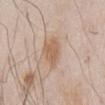Q: Was a biopsy performed?
A: catalogued during a skin exam; not biopsied
Q: What did automated image analysis measure?
A: an average lesion color of about L≈60 a*≈18 b*≈31 (CIELAB), roughly 8 lightness units darker than nearby skin, and a normalized border contrast of about 6.5; an automated nevus-likeness rating near 60 out of 100 and a detector confidence of about 100 out of 100 that the crop contains a lesion
Q: What is the lesion's diameter?
A: about 3.5 mm
Q: What are the patient's age and sex?
A: male, about 80 years old
Q: How was the tile lit?
A: white-light illumination
Q: How was this image acquired?
A: 15 mm crop, total-body photography
Q: What is the anatomic site?
A: the chest